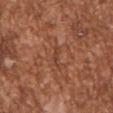Part of a total-body skin-imaging series; this lesion was reviewed on a skin check and was not flagged for biopsy.
A 15 mm crop from a total-body photograph taken for skin-cancer surveillance.
Longest diameter approximately 2.5 mm.
The subject is a male aged 43 to 47.
On the arm.